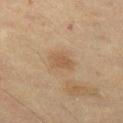Q: Was this lesion biopsied?
A: imaged on a skin check; not biopsied
Q: Illumination type?
A: cross-polarized
Q: Patient demographics?
A: female, in their mid-50s
Q: How was this image acquired?
A: ~15 mm tile from a whole-body skin photo
Q: What is the anatomic site?
A: the left thigh
Q: What is the lesion's diameter?
A: ≈3 mm